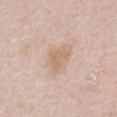Assessment:
No biopsy was performed on this lesion — it was imaged during a full skin examination and was not determined to be concerning.
Image and clinical context:
The recorded lesion diameter is about 3.5 mm. The patient is a female aged approximately 60. The tile uses white-light illumination. Cropped from a whole-body photographic skin survey; the tile spans about 15 mm. Located on the abdomen.